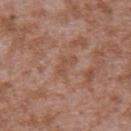biopsy_status: not biopsied; imaged during a skin examination
patient:
  sex: male
  age_approx: 45
image:
  source: total-body photography crop
  field_of_view_mm: 15
lighting: white-light
site: upper back
lesion_size:
  long_diameter_mm_approx: 3.0